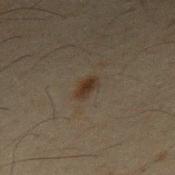| feature | finding |
|---|---|
| biopsy status | catalogued during a skin exam; not biopsied |
| lighting | cross-polarized illumination |
| subject | male, aged approximately 65 |
| imaging modality | ~15 mm tile from a whole-body skin photo |
| diameter | ~3 mm (longest diameter) |
| site | the chest |